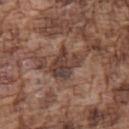Q: Was this lesion biopsied?
A: catalogued during a skin exam; not biopsied
Q: Illumination type?
A: white-light illumination
Q: What is the lesion's diameter?
A: ≈4 mm
Q: Lesion location?
A: the arm
Q: How was this image acquired?
A: ~15 mm crop, total-body skin-cancer survey
Q: Who is the patient?
A: male, approximately 75 years of age
Q: What did automated image analysis measure?
A: a lesion color around L≈39 a*≈18 b*≈23 in CIELAB and a lesion–skin lightness drop of about 10; a border-irregularity index near 4/10, a color-variation rating of about 6.5/10, and peripheral color asymmetry of about 2; a classifier nevus-likeness of about 0/100 and lesion-presence confidence of about 55/100